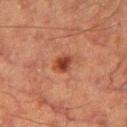The lesion was tiled from a total-body skin photograph and was not biopsied.
The lesion-visualizer software estimated about 10 CIELAB-L* units darker than the surrounding skin and a normalized border contrast of about 9. The analysis additionally found a color-variation rating of about 3.5/10 and a peripheral color-asymmetry measure near 1.
Imaged with cross-polarized lighting.
A close-up tile cropped from a whole-body skin photograph, about 15 mm across.
The lesion is on the left thigh.
About 2.5 mm across.
A male subject aged around 65.